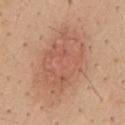{
  "image": {
    "source": "total-body photography crop",
    "field_of_view_mm": 15
  },
  "site": "mid back",
  "automated_metrics": {
    "vs_skin_darker_L": 8.0,
    "vs_skin_contrast_norm": 5.5,
    "color_variation_0_10": 4.0,
    "peripheral_color_asymmetry": 1.5
  },
  "patient": {
    "sex": "male",
    "age_approx": 40
  }
}